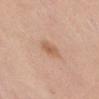Clinical impression:
Recorded during total-body skin imaging; not selected for excision or biopsy.
Clinical summary:
A female subject approximately 50 years of age. The lesion-visualizer software estimated border irregularity of about 2 on a 0–10 scale. A close-up tile cropped from a whole-body skin photograph, about 15 mm across. Located on the front of the torso.